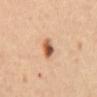Clinical impression: The lesion was photographed on a routine skin check and not biopsied; there is no pathology result. Background: The lesion is located on the back. A region of skin cropped from a whole-body photographic capture, roughly 15 mm wide. The patient is a female approximately 45 years of age.